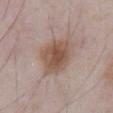biopsy status: imaged on a skin check; not biopsied
location: the abdomen
image-analysis metrics: a footprint of about 15 mm², an outline eccentricity of about 0.5 (0 = round, 1 = elongated), and a shape-asymmetry score of about 0.2 (0 = symmetric); an average lesion color of about L≈52 a*≈17 b*≈26 (CIELAB), a lesion–skin lightness drop of about 11, and a lesion-to-skin contrast of about 8.5 (normalized; higher = more distinct); a border-irregularity rating of about 2/10, a within-lesion color-variation index near 4.5/10, and peripheral color asymmetry of about 1
illumination: white-light illumination
acquisition: ~15 mm tile from a whole-body skin photo
patient: male, roughly 55 years of age
size: about 4.5 mm